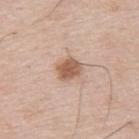Clinical impression:
Recorded during total-body skin imaging; not selected for excision or biopsy.
Clinical summary:
On the upper back. Measured at roughly 3 mm in maximum diameter. A male patient aged 73 to 77. A 15 mm close-up extracted from a 3D total-body photography capture. The tile uses white-light illumination.